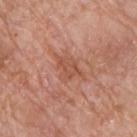No biopsy was performed on this lesion — it was imaged during a full skin examination and was not determined to be concerning. The lesion is located on the chest. A male subject roughly 75 years of age. The recorded lesion diameter is about 4 mm. Automated image analysis of the tile measured a color-variation rating of about 1.5/10 and peripheral color asymmetry of about 0.5. The analysis additionally found an automated nevus-likeness rating near 0 out of 100 and a lesion-detection confidence of about 100/100. Imaged with white-light lighting. This image is a 15 mm lesion crop taken from a total-body photograph.